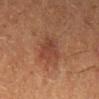Acquisition and patient details: From the left lower leg. The subject is a female aged approximately 50. A lesion tile, about 15 mm wide, cut from a 3D total-body photograph.